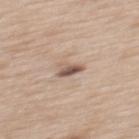Part of a total-body skin-imaging series; this lesion was reviewed on a skin check and was not flagged for biopsy.
From the mid back.
The tile uses white-light illumination.
A 15 mm close-up tile from a total-body photography series done for melanoma screening.
A female patient, about 40 years old.
About 2.5 mm across.